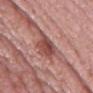Q: Is there a histopathology result?
A: imaged on a skin check; not biopsied
Q: Patient demographics?
A: female, in their 40s
Q: What kind of image is this?
A: 15 mm crop, total-body photography
Q: How was the tile lit?
A: white-light illumination
Q: What is the anatomic site?
A: the leg